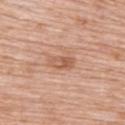{
  "biopsy_status": "not biopsied; imaged during a skin examination",
  "lesion_size": {
    "long_diameter_mm_approx": 3.5
  },
  "site": "upper back",
  "image": {
    "source": "total-body photography crop",
    "field_of_view_mm": 15
  },
  "patient": {
    "sex": "female",
    "age_approx": 70
  },
  "automated_metrics": {
    "area_mm2_approx": 5.5,
    "eccentricity": 0.85,
    "cielab_L": 58,
    "cielab_a": 23,
    "cielab_b": 32,
    "vs_skin_darker_L": 9.0,
    "vs_skin_contrast_norm": 6.5,
    "border_irregularity_0_10": 2.0,
    "color_variation_0_10": 5.5,
    "peripheral_color_asymmetry": 2.0,
    "lesion_detection_confidence_0_100": 100
  },
  "lighting": "white-light"
}